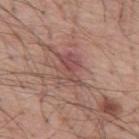– biopsy status · imaged on a skin check; not biopsied
– image · total-body-photography crop, ~15 mm field of view
– illumination · white-light
– patient · male, approximately 55 years of age
– body site · the mid back
– diameter · about 4 mm